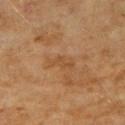follow-up: total-body-photography surveillance lesion; no biopsy
anatomic site: the arm
imaging modality: 15 mm crop, total-body photography
patient: female, about 60 years old